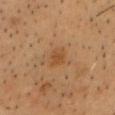Part of a total-body skin-imaging series; this lesion was reviewed on a skin check and was not flagged for biopsy.
On the head or neck.
A 15 mm close-up extracted from a 3D total-body photography capture.
Approximately 2.5 mm at its widest.
The tile uses cross-polarized illumination.
A male patient, aged 58–62.